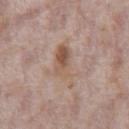Notes:
- anatomic site — the abdomen
- image — total-body-photography crop, ~15 mm field of view
- lesion diameter — ≈5 mm
- subject — male, approximately 70 years of age